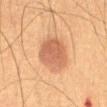| feature | finding |
|---|---|
| follow-up | catalogued during a skin exam; not biopsied |
| acquisition | 15 mm crop, total-body photography |
| lighting | cross-polarized illumination |
| image-analysis metrics | a lesion area of about 15 mm² and two-axis asymmetry of about 0.15; a mean CIELAB color near L≈49 a*≈20 b*≈30; border irregularity of about 2 on a 0–10 scale and a peripheral color-asymmetry measure near 1 |
| location | the abdomen |
| lesion diameter | ~5 mm (longest diameter) |
| patient | male, roughly 50 years of age |